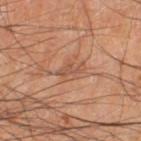<case>
<site>right thigh</site>
<patient>
  <sex>male</sex>
  <age_approx>65</age_approx>
</patient>
<lesion_size>
  <long_diameter_mm_approx>3.0</long_diameter_mm_approx>
</lesion_size>
<image>
  <source>total-body photography crop</source>
  <field_of_view_mm>15</field_of_view_mm>
</image>
<lighting>cross-polarized</lighting>
</case>